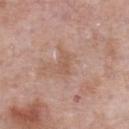biopsy status: no biopsy performed (imaged during a skin exam)
body site: the chest
lesion size: ~2.5 mm (longest diameter)
patient: male, aged 53–57
acquisition: ~15 mm crop, total-body skin-cancer survey